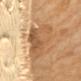<case>
<biopsy_status>not biopsied; imaged during a skin examination</biopsy_status>
<patient>
  <sex>female</sex>
  <age_approx>65</age_approx>
</patient>
<lighting>cross-polarized</lighting>
<site>mid back</site>
<image>
  <source>total-body photography crop</source>
  <field_of_view_mm>15</field_of_view_mm>
</image>
<lesion_size>
  <long_diameter_mm_approx>7.0</long_diameter_mm_approx>
</lesion_size>
</case>